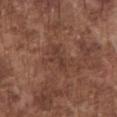size=≈3.5 mm | illumination=white-light illumination | patient=male, approximately 75 years of age | image source=15 mm crop, total-body photography | site=the chest.